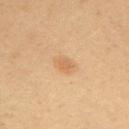On the arm. A female subject in their 40s. A 15 mm close-up tile from a total-body photography series done for melanoma screening.